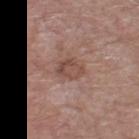Impression:
No biopsy was performed on this lesion — it was imaged during a full skin examination and was not determined to be concerning.
Background:
The tile uses white-light illumination. Measured at roughly 3 mm in maximum diameter. Cropped from a total-body skin-imaging series; the visible field is about 15 mm. A female patient aged 53 to 57. From the left thigh.